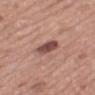Case summary:
• notes · no biopsy performed (imaged during a skin exam)
• patient · male, roughly 75 years of age
• site · the back
• size · ≈3 mm
• imaging modality · ~15 mm tile from a whole-body skin photo